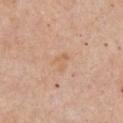Captured during whole-body skin photography for melanoma surveillance; the lesion was not biopsied. A male subject, aged 53–57. About 2.5 mm across. A lesion tile, about 15 mm wide, cut from a 3D total-body photograph. Located on the chest. Automated tile analysis of the lesion measured roughly 5 lightness units darker than nearby skin and a lesion-to-skin contrast of about 5 (normalized; higher = more distinct).